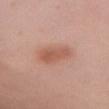<lesion>
  <biopsy_status>not biopsied; imaged during a skin examination</biopsy_status>
  <image>
    <source>total-body photography crop</source>
    <field_of_view_mm>15</field_of_view_mm>
  </image>
  <patient>
    <sex>female</sex>
    <age_approx>25</age_approx>
  </patient>
  <site>chest</site>
</lesion>